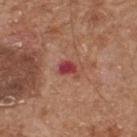follow-up = total-body-photography surveillance lesion; no biopsy | image source = ~15 mm crop, total-body skin-cancer survey | patient = male, aged approximately 65 | location = the upper back | lighting = white-light illumination.